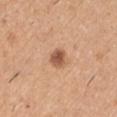biopsy_status: not biopsied; imaged during a skin examination
lesion_size:
  long_diameter_mm_approx: 2.5
site: right upper arm
image:
  source: total-body photography crop
  field_of_view_mm: 15
lighting: white-light
patient:
  sex: male
  age_approx: 40
automated_metrics:
  shape_asymmetry: 0.2
  cielab_L: 55
  cielab_a: 23
  cielab_b: 33
  vs_skin_darker_L: 14.0
  vs_skin_contrast_norm: 9.0
  color_variation_0_10: 3.5
  peripheral_color_asymmetry: 1.5